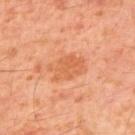Part of a total-body skin-imaging series; this lesion was reviewed on a skin check and was not flagged for biopsy.
A close-up tile cropped from a whole-body skin photograph, about 15 mm across.
About 4 mm across.
The tile uses cross-polarized illumination.
A male subject, roughly 60 years of age.
The lesion is on the upper back.